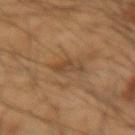Case summary:
- workup · no biopsy performed (imaged during a skin exam)
- lesion size · ~3.5 mm (longest diameter)
- tile lighting · cross-polarized illumination
- automated lesion analysis · a footprint of about 4 mm², an outline eccentricity of about 0.95 (0 = round, 1 = elongated), and two-axis asymmetry of about 0.4; a lesion color around L≈45 a*≈18 b*≈33 in CIELAB, about 8 CIELAB-L* units darker than the surrounding skin, and a normalized lesion–skin contrast near 6; a nevus-likeness score of about 0/100 and a detector confidence of about 80 out of 100 that the crop contains a lesion
- image source · ~15 mm crop, total-body skin-cancer survey
- anatomic site · the right forearm
- subject · male, in their mid- to late 60s